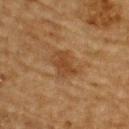acquisition = ~15 mm crop, total-body skin-cancer survey
subject = male, aged 83 or older
body site = the upper back
tile lighting = cross-polarized illumination
lesion diameter = ~3.5 mm (longest diameter)